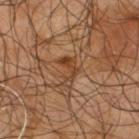Part of a total-body skin-imaging series; this lesion was reviewed on a skin check and was not flagged for biopsy.
Located on the back.
Captured under cross-polarized illumination.
A close-up tile cropped from a whole-body skin photograph, about 15 mm across.
The subject is a male in their mid- to late 60s.